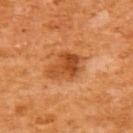Q: What is the lesion's diameter?
A: about 4 mm
Q: Who is the patient?
A: female, about 55 years old
Q: What lighting was used for the tile?
A: cross-polarized illumination
Q: What is the imaging modality?
A: 15 mm crop, total-body photography
Q: Lesion location?
A: the back